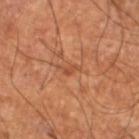follow-up = imaged on a skin check; not biopsied | subject = male, aged 58–62 | diameter = ≈2.5 mm | illumination = cross-polarized | body site = the left lower leg | image = ~15 mm crop, total-body skin-cancer survey.